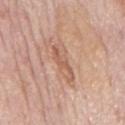This lesion was catalogued during total-body skin photography and was not selected for biopsy.
Cropped from a total-body skin-imaging series; the visible field is about 15 mm.
Captured under white-light illumination.
An algorithmic analysis of the crop reported a footprint of about 5.5 mm², a shape eccentricity near 0.95, and a shape-asymmetry score of about 0.4 (0 = symmetric). The analysis additionally found an average lesion color of about L≈59 a*≈22 b*≈30 (CIELAB), roughly 9 lightness units darker than nearby skin, and a lesion-to-skin contrast of about 6 (normalized; higher = more distinct). It also reported an automated nevus-likeness rating near 0 out of 100.
The lesion is located on the mid back.
A male subject, about 75 years old.
Approximately 4.5 mm at its widest.